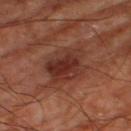Part of a total-body skin-imaging series; this lesion was reviewed on a skin check and was not flagged for biopsy.
The lesion is located on the right thigh.
About 5.5 mm across.
A 15 mm close-up extracted from a 3D total-body photography capture.
A subject aged 63 to 67.
Captured under cross-polarized illumination.